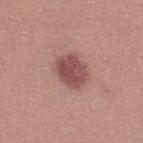– workup — catalogued during a skin exam; not biopsied
– imaging modality — ~15 mm tile from a whole-body skin photo
– patient — female, in their mid-30s
– site — the left leg